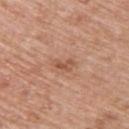Clinical impression:
Imaged during a routine full-body skin examination; the lesion was not biopsied and no histopathology is available.
Image and clinical context:
A 15 mm close-up tile from a total-body photography series done for melanoma screening. Measured at roughly 2.5 mm in maximum diameter. The total-body-photography lesion software estimated an outline eccentricity of about 0.85 (0 = round, 1 = elongated) and a symmetry-axis asymmetry near 0.35. And it measured a normalized lesion–skin contrast near 6.5. The patient is a male aged around 60. On the upper back.